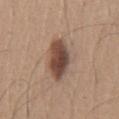notes: imaged on a skin check; not biopsied | image: 15 mm crop, total-body photography | tile lighting: white-light illumination | body site: the chest | patient: male, about 65 years old.